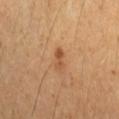This lesion was catalogued during total-body skin photography and was not selected for biopsy.
From the left forearm.
A female subject about 45 years old.
Cropped from a total-body skin-imaging series; the visible field is about 15 mm.